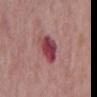Imaged during a routine full-body skin examination; the lesion was not biopsied and no histopathology is available.
Automated image analysis of the tile measured a mean CIELAB color near L≈43 a*≈32 b*≈18 and a normalized lesion–skin contrast near 10.5. It also reported a border-irregularity rating of about 2/10, internal color variation of about 5.5 on a 0–10 scale, and a peripheral color-asymmetry measure near 1.5. It also reported an automated nevus-likeness rating near 0 out of 100 and a lesion-detection confidence of about 100/100.
The lesion's longest dimension is about 3.5 mm.
A male patient, approximately 65 years of age.
The tile uses white-light illumination.
A 15 mm crop from a total-body photograph taken for skin-cancer surveillance.
The lesion is located on the mid back.